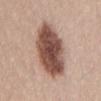Assessment: Part of a total-body skin-imaging series; this lesion was reviewed on a skin check and was not flagged for biopsy. Clinical summary: This image is a 15 mm lesion crop taken from a total-body photograph. A female patient, about 20 years old. On the lower back. Captured under white-light illumination. The recorded lesion diameter is about 6.5 mm.